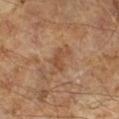Q: Was a biopsy performed?
A: total-body-photography surveillance lesion; no biopsy
Q: Lesion size?
A: ~4 mm (longest diameter)
Q: Patient demographics?
A: male, about 65 years old
Q: What is the imaging modality?
A: ~15 mm crop, total-body skin-cancer survey
Q: How was the tile lit?
A: cross-polarized illumination
Q: What did automated image analysis measure?
A: an automated nevus-likeness rating near 0 out of 100 and a lesion-detection confidence of about 100/100